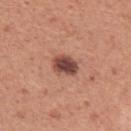No biopsy was performed on this lesion — it was imaged during a full skin examination and was not determined to be concerning. A female subject, aged approximately 55. Automated tile analysis of the lesion measured a footprint of about 6 mm². The lesion is on the left upper arm. The lesion's longest dimension is about 3 mm. A lesion tile, about 15 mm wide, cut from a 3D total-body photograph.